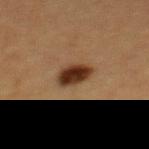– diameter — about 3.5 mm
– patient — female, aged approximately 40
– image — total-body-photography crop, ~15 mm field of view
– location — the back